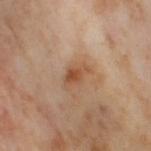biopsy status: imaged on a skin check; not biopsied
image source: ~15 mm crop, total-body skin-cancer survey
TBP lesion metrics: an average lesion color of about L≈53 a*≈20 b*≈33 (CIELAB) and a lesion–skin lightness drop of about 9; an automated nevus-likeness rating near 5 out of 100 and a detector confidence of about 100 out of 100 that the crop contains a lesion
size: ≈3.5 mm
subject: female, about 55 years old
tile lighting: cross-polarized
anatomic site: the right thigh